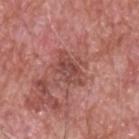Assessment:
Part of a total-body skin-imaging series; this lesion was reviewed on a skin check and was not flagged for biopsy.
Image and clinical context:
A 15 mm close-up tile from a total-body photography series done for melanoma screening. A male subject aged 58 to 62. Imaged with white-light lighting. The lesion is on the upper back.